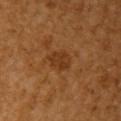follow-up: imaged on a skin check; not biopsied | acquisition: ~15 mm tile from a whole-body skin photo | subject: female, about 55 years old | anatomic site: the right upper arm | lighting: cross-polarized illumination.